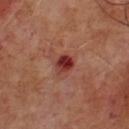{"biopsy_status": "not biopsied; imaged during a skin examination", "patient": {"sex": "male", "age_approx": 65}, "lighting": "cross-polarized", "image": {"source": "total-body photography crop", "field_of_view_mm": 15}, "lesion_size": {"long_diameter_mm_approx": 2.5}}